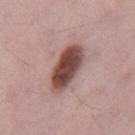The lesion is on the mid back.
A 15 mm close-up tile from a total-body photography series done for melanoma screening.
Automated tile analysis of the lesion measured an average lesion color of about L≈47 a*≈21 b*≈22 (CIELAB) and roughly 18 lightness units darker than nearby skin.
The lesion's longest dimension is about 5.5 mm.
The patient is a male aged approximately 70.